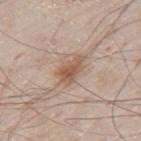Clinical impression:
Captured during whole-body skin photography for melanoma surveillance; the lesion was not biopsied.
Background:
The lesion is on the mid back. Approximately 3.5 mm at its widest. A roughly 15 mm field-of-view crop from a total-body skin photograph. The tile uses white-light illumination. The patient is a male aged around 80. The lesion-visualizer software estimated a lesion area of about 6 mm². And it measured a mean CIELAB color near L≈56 a*≈18 b*≈29, a lesion–skin lightness drop of about 10, and a normalized lesion–skin contrast near 7.5. And it measured a border-irregularity index near 3.5/10, a color-variation rating of about 3.5/10, and radial color variation of about 1.